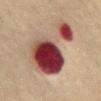notes = imaged on a skin check; not biopsied
subject = male, aged around 75
lesion size = ~9.5 mm (longest diameter)
tile lighting = cross-polarized
image source = 15 mm crop, total-body photography
site = the chest